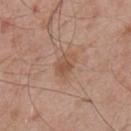<case>
  <biopsy_status>not biopsied; imaged during a skin examination</biopsy_status>
  <lesion_size>
    <long_diameter_mm_approx>3.5</long_diameter_mm_approx>
  </lesion_size>
  <lighting>white-light</lighting>
  <image>
    <source>total-body photography crop</source>
    <field_of_view_mm>15</field_of_view_mm>
  </image>
  <site>mid back</site>
  <patient>
    <sex>male</sex>
    <age_approx>55</age_approx>
  </patient>
</case>